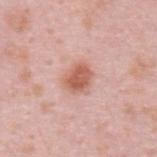  biopsy_status: not biopsied; imaged during a skin examination
  site: upper back
  patient:
    sex: male
    age_approx: 35
  automated_metrics:
    cielab_L: 60
    cielab_a: 25
    cielab_b: 28
    vs_skin_darker_L: 12.0
    vs_skin_contrast_norm: 8.0
    border_irregularity_0_10: 2.0
    color_variation_0_10: 3.0
    peripheral_color_asymmetry: 1.0
    nevus_likeness_0_100: 100
    lesion_detection_confidence_0_100: 100
  lesion_size:
    long_diameter_mm_approx: 3.5
  image:
    source: total-body photography crop
    field_of_view_mm: 15